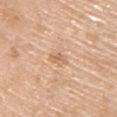notes: total-body-photography surveillance lesion; no biopsy
subject: male, about 70 years old
image source: ~15 mm tile from a whole-body skin photo
lesion size: ≈3 mm
lighting: white-light
site: the back